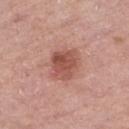Image and clinical context: From the right lower leg. A male patient aged around 75. This is a white-light tile. This image is a 15 mm lesion crop taken from a total-body photograph. The recorded lesion diameter is about 4 mm. The lesion-visualizer software estimated an area of roughly 12 mm², an eccentricity of roughly 0.55, and two-axis asymmetry of about 0.15. And it measured a border-irregularity index near 1.5/10 and radial color variation of about 1.5.